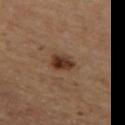{"patient": {"sex": "male", "age_approx": 65}, "site": "leg", "image": {"source": "total-body photography crop", "field_of_view_mm": 15}, "automated_metrics": {"cielab_L": 35, "cielab_a": 19, "cielab_b": 29, "vs_skin_darker_L": 12.0, "vs_skin_contrast_norm": 10.5, "color_variation_0_10": 5.0, "peripheral_color_asymmetry": 2.0}}